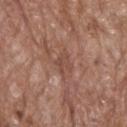No biopsy was performed on this lesion — it was imaged during a full skin examination and was not determined to be concerning. This is a white-light tile. A male patient, aged 68–72. A 15 mm crop from a total-body photograph taken for skin-cancer surveillance. From the upper back. Approximately 2.5 mm at its widest.